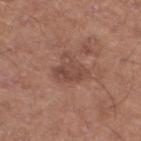{"biopsy_status": "not biopsied; imaged during a skin examination", "image": {"source": "total-body photography crop", "field_of_view_mm": 15}, "patient": {"sex": "male", "age_approx": 50}, "site": "leg", "lesion_size": {"long_diameter_mm_approx": 4.5}, "lighting": "white-light"}